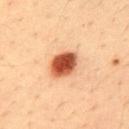notes = total-body-photography surveillance lesion; no biopsy
lighting = cross-polarized illumination
patient = male, aged 33–37
location = the upper back
lesion diameter = about 4 mm
imaging modality = ~15 mm tile from a whole-body skin photo